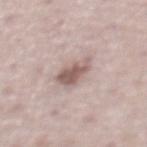Context:
The lesion-visualizer software estimated an automated nevus-likeness rating near 40 out of 100 and lesion-presence confidence of about 95/100. Imaged with white-light lighting. The lesion is on the abdomen. A 15 mm close-up extracted from a 3D total-body photography capture. A male patient in their mid-70s. Approximately 3.5 mm at its widest.